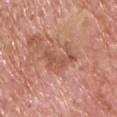Q: Was a biopsy performed?
A: imaged on a skin check; not biopsied
Q: What kind of image is this?
A: ~15 mm crop, total-body skin-cancer survey
Q: How large is the lesion?
A: ~5 mm (longest diameter)
Q: What are the patient's age and sex?
A: male, in their mid-70s
Q: Automated lesion metrics?
A: a footprint of about 9 mm², an eccentricity of roughly 0.8, and two-axis asymmetry of about 0.6; a lesion color around L≈54 a*≈25 b*≈31 in CIELAB, about 8 CIELAB-L* units darker than the surrounding skin, and a normalized border contrast of about 6
Q: Where on the body is the lesion?
A: the chest
Q: How was the tile lit?
A: white-light illumination